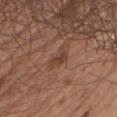Q: How large is the lesion?
A: ≈3 mm
Q: Lesion location?
A: the left upper arm
Q: What are the patient's age and sex?
A: male, in their mid-50s
Q: What is the imaging modality?
A: total-body-photography crop, ~15 mm field of view